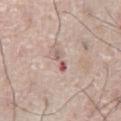This lesion was catalogued during total-body skin photography and was not selected for biopsy. The lesion is on the abdomen. An algorithmic analysis of the crop reported an area of roughly 3 mm² and an eccentricity of roughly 0.95. It also reported about 11 CIELAB-L* units darker than the surrounding skin and a normalized border contrast of about 7.5. The analysis additionally found an automated nevus-likeness rating near 0 out of 100. A male subject approximately 75 years of age. This image is a 15 mm lesion crop taken from a total-body photograph.